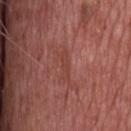Clinical impression: Captured during whole-body skin photography for melanoma surveillance; the lesion was not biopsied. Context: A male subject, approximately 75 years of age. On the head or neck. A close-up tile cropped from a whole-body skin photograph, about 15 mm across.